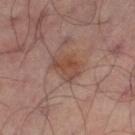The lesion was tiled from a total-body skin photograph and was not biopsied. Located on the left thigh. A lesion tile, about 15 mm wide, cut from a 3D total-body photograph. A male patient aged around 65.